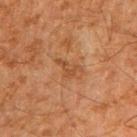notes — total-body-photography surveillance lesion; no biopsy | lesion size — ~3 mm (longest diameter) | subject — male, in their mid-60s | automated lesion analysis — an outline eccentricity of about 0.8 (0 = round, 1 = elongated) and two-axis asymmetry of about 0.6; border irregularity of about 6.5 on a 0–10 scale, internal color variation of about 1 on a 0–10 scale, and peripheral color asymmetry of about 0.5 | location — the right upper arm | imaging modality — ~15 mm tile from a whole-body skin photo | tile lighting — cross-polarized illumination.